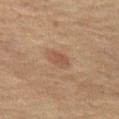| feature | finding |
|---|---|
| follow-up | imaged on a skin check; not biopsied |
| size | ≈2.5 mm |
| lighting | cross-polarized |
| imaging modality | ~15 mm tile from a whole-body skin photo |
| automated metrics | a footprint of about 4 mm² and two-axis asymmetry of about 0.2; an average lesion color of about L≈43 a*≈18 b*≈25 (CIELAB), a lesion–skin lightness drop of about 7, and a normalized lesion–skin contrast near 5.5; a border-irregularity rating of about 2/10, a within-lesion color-variation index near 1.5/10, and radial color variation of about 0.5; a classifier nevus-likeness of about 30/100 and a detector confidence of about 100 out of 100 that the crop contains a lesion |
| subject | female, aged approximately 65 |
| body site | the left thigh |